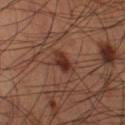  patient:
    sex: male
    age_approx: 60
  site: left thigh
  automated_metrics:
    border_irregularity_0_10: 3.5
    color_variation_0_10: 4.5
    peripheral_color_asymmetry: 1.5
    lesion_detection_confidence_0_100: 100
  lesion_size:
    long_diameter_mm_approx: 3.5
  image:
    source: total-body photography crop
    field_of_view_mm: 15
  lighting: cross-polarized